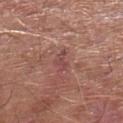Imaged during a routine full-body skin examination; the lesion was not biopsied and no histopathology is available. About 2.5 mm across. On the left lower leg. A male patient approximately 65 years of age. Captured under white-light illumination. Cropped from a total-body skin-imaging series; the visible field is about 15 mm.